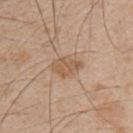Acquisition and patient details:
A 15 mm close-up extracted from a 3D total-body photography capture. Longest diameter approximately 4 mm. Automated tile analysis of the lesion measured a lesion area of about 7.5 mm² and a shape eccentricity near 0.75. A male patient aged 48–52. This is a white-light tile. The lesion is located on the chest.